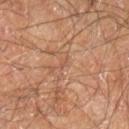This lesion was catalogued during total-body skin photography and was not selected for biopsy.
Cropped from a whole-body photographic skin survey; the tile spans about 15 mm.
A male patient, in their 60s.
Located on the right lower leg.
Automated tile analysis of the lesion measured a footprint of about 0.5 mm².
The lesion's longest dimension is about 1 mm.
The tile uses cross-polarized illumination.